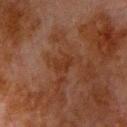Q: Is there a histopathology result?
A: catalogued during a skin exam; not biopsied
Q: What did automated image analysis measure?
A: an area of roughly 4 mm², a shape eccentricity near 0.8, and a symmetry-axis asymmetry near 0.35; border irregularity of about 3.5 on a 0–10 scale and a within-lesion color-variation index near 1.5/10; an automated nevus-likeness rating near 0 out of 100 and a detector confidence of about 100 out of 100 that the crop contains a lesion
Q: How was this image acquired?
A: ~15 mm tile from a whole-body skin photo
Q: What lighting was used for the tile?
A: cross-polarized
Q: What are the patient's age and sex?
A: male, aged around 80
Q: Lesion size?
A: ~2.5 mm (longest diameter)
Q: Where on the body is the lesion?
A: the front of the torso